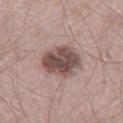workup = total-body-photography surveillance lesion; no biopsy
image source = 15 mm crop, total-body photography
automated metrics = a footprint of about 15 mm², a shape eccentricity near 0.6, and two-axis asymmetry of about 0.2; a mean CIELAB color near L≈49 a*≈17 b*≈20, roughly 15 lightness units darker than nearby skin, and a normalized border contrast of about 10.5
site = the left thigh
lighting = white-light illumination
patient = male, about 60 years old
lesion diameter = about 5 mm